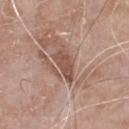Impression:
No biopsy was performed on this lesion — it was imaged during a full skin examination and was not determined to be concerning.
Background:
The lesion is on the chest. The subject is a male about 65 years old. The recorded lesion diameter is about 4 mm. A 15 mm close-up extracted from a 3D total-body photography capture.